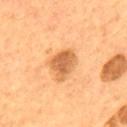Case summary:
- notes: imaged on a skin check; not biopsied
- lesion size: about 3.5 mm
- image-analysis metrics: a footprint of about 8 mm² and two-axis asymmetry of about 0.3; a mean CIELAB color near L≈58 a*≈25 b*≈41, a lesion–skin lightness drop of about 13, and a normalized lesion–skin contrast near 8; border irregularity of about 2.5 on a 0–10 scale and internal color variation of about 3 on a 0–10 scale
- subject: male, roughly 55 years of age
- tile lighting: cross-polarized
- imaging modality: ~15 mm crop, total-body skin-cancer survey
- location: the upper back